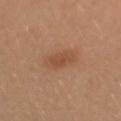Q: Is there a histopathology result?
A: no biopsy performed (imaged during a skin exam)